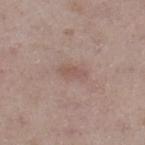Recorded during total-body skin imaging; not selected for excision or biopsy. The lesion is on the leg. A close-up tile cropped from a whole-body skin photograph, about 15 mm across. The subject is a male approximately 60 years of age. Captured under white-light illumination. Automated image analysis of the tile measured a mean CIELAB color near L≈53 a*≈18 b*≈23, a lesion–skin lightness drop of about 7, and a normalized border contrast of about 5.5. The analysis additionally found a classifier nevus-likeness of about 0/100 and a detector confidence of about 100 out of 100 that the crop contains a lesion.